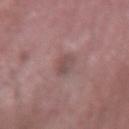The lesion is on the right forearm. A 15 mm close-up extracted from a 3D total-body photography capture. A male patient aged approximately 65. Longest diameter approximately 2.5 mm. This is a white-light tile.